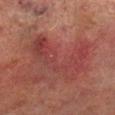The lesion was tiled from a total-body skin photograph and was not biopsied. Automated image analysis of the tile measured an area of roughly 18 mm², a shape eccentricity near 0.9, and a shape-asymmetry score of about 0.55 (0 = symmetric). It also reported a classifier nevus-likeness of about 0/100 and a detector confidence of about 95 out of 100 that the crop contains a lesion. A male patient, aged around 75. A close-up tile cropped from a whole-body skin photograph, about 15 mm across. About 8 mm across. The lesion is on the right lower leg.